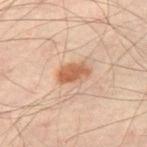  biopsy_status: not biopsied; imaged during a skin examination
  site: left thigh
  lesion_size:
    long_diameter_mm_approx: 3.5
  lighting: cross-polarized
  patient:
    sex: male
    age_approx: 50
  image:
    source: total-body photography crop
    field_of_view_mm: 15
  automated_metrics:
    cielab_L: 48
    cielab_a: 20
    cielab_b: 29
    vs_skin_darker_L: 10.0
    vs_skin_contrast_norm: 8.5
    border_irregularity_0_10: 2.0
    color_variation_0_10: 2.5
    peripheral_color_asymmetry: 1.0
    lesion_detection_confidence_0_100: 100